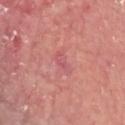Imaged during a routine full-body skin examination; the lesion was not biopsied and no histopathology is available. The lesion is on the head or neck. This image is a 15 mm lesion crop taken from a total-body photograph. This is a white-light tile. The patient is a male aged 63 to 67.